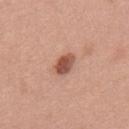Impression:
Part of a total-body skin-imaging series; this lesion was reviewed on a skin check and was not flagged for biopsy.
Clinical summary:
A 15 mm crop from a total-body photograph taken for skin-cancer surveillance. Measured at roughly 3 mm in maximum diameter. Captured under white-light illumination. The lesion is located on the upper back. The lesion-visualizer software estimated a lesion–skin lightness drop of about 15 and a normalized lesion–skin contrast near 10. It also reported border irregularity of about 2 on a 0–10 scale and a within-lesion color-variation index near 3.5/10. The analysis additionally found a classifier nevus-likeness of about 85/100 and a detector confidence of about 100 out of 100 that the crop contains a lesion. A female patient, aged 38 to 42.